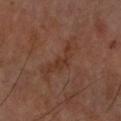{
  "biopsy_status": "not biopsied; imaged during a skin examination",
  "automated_metrics": {
    "area_mm2_approx": 9.5,
    "border_irregularity_0_10": 7.5,
    "color_variation_0_10": 2.5,
    "lesion_detection_confidence_0_100": 100
  },
  "lighting": "cross-polarized",
  "patient": {
    "sex": "male",
    "age_approx": 70
  },
  "image": {
    "source": "total-body photography crop",
    "field_of_view_mm": 15
  },
  "site": "right forearm",
  "lesion_size": {
    "long_diameter_mm_approx": 6.5
  }
}